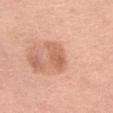notes = catalogued during a skin exam; not biopsied | lighting = white-light | lesion size = about 4 mm | image = ~15 mm crop, total-body skin-cancer survey | location = the left thigh | subject = female, aged around 65.